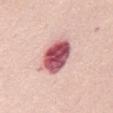biopsy_status: not biopsied; imaged during a skin examination
patient:
  sex: female
  age_approx: 65
automated_metrics:
  vs_skin_darker_L: 22.0
  vs_skin_contrast_norm: 13.5
  border_irregularity_0_10: 1.5
  peripheral_color_asymmetry: 2.5
image:
  source: total-body photography crop
  field_of_view_mm: 15
lighting: white-light
site: mid back
lesion_size:
  long_diameter_mm_approx: 5.0